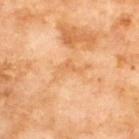site: upper back
lesion_size:
  long_diameter_mm_approx: 4.5
patient:
  sex: male
  age_approx: 70
lighting: cross-polarized
automated_metrics:
  border_irregularity_0_10: 8.0
  color_variation_0_10: 2.0
  nevus_likeness_0_100: 0
  lesion_detection_confidence_0_100: 100
image:
  source: total-body photography crop
  field_of_view_mm: 15